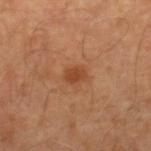<record>
  <biopsy_status>not biopsied; imaged during a skin examination</biopsy_status>
  <lighting>cross-polarized</lighting>
  <lesion_size>
    <long_diameter_mm_approx>2.0</long_diameter_mm_approx>
  </lesion_size>
  <patient>
    <sex>male</sex>
    <age_approx>55</age_approx>
  </patient>
  <automated_metrics>
    <eccentricity>0.7</eccentricity>
    <shape_asymmetry>0.2</shape_asymmetry>
    <border_irregularity_0_10>2.0</border_irregularity_0_10>
    <color_variation_0_10>1.0</color_variation_0_10>
    <peripheral_color_asymmetry>0.5</peripheral_color_asymmetry>
    <nevus_likeness_0_100>85</nevus_likeness_0_100>
    <lesion_detection_confidence_0_100>100</lesion_detection_confidence_0_100>
  </automated_metrics>
  <image>
    <source>total-body photography crop</source>
    <field_of_view_mm>15</field_of_view_mm>
  </image>
  <site>right lower leg</site>
</record>